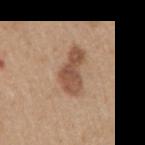Captured during whole-body skin photography for melanoma surveillance; the lesion was not biopsied. Located on the right upper arm. A lesion tile, about 15 mm wide, cut from a 3D total-body photograph. A male patient, approximately 55 years of age.